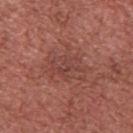  biopsy_status: not biopsied; imaged during a skin examination
  automated_metrics:
    area_mm2_approx: 7.5
    eccentricity: 0.75
    nevus_likeness_0_100: 0
    lesion_detection_confidence_0_100: 100
  lesion_size:
    long_diameter_mm_approx: 4.0
  patient:
    sex: male
    age_approx: 45
  site: chest
  image:
    source: total-body photography crop
    field_of_view_mm: 15